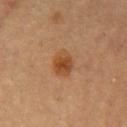The lesion's longest dimension is about 3.5 mm.
A female patient, aged around 60.
On the chest.
Automated tile analysis of the lesion measured an average lesion color of about L≈41 a*≈20 b*≈34 (CIELAB), roughly 9 lightness units darker than nearby skin, and a normalized lesion–skin contrast near 8.5. And it measured border irregularity of about 1.5 on a 0–10 scale, internal color variation of about 4 on a 0–10 scale, and a peripheral color-asymmetry measure near 1. And it measured a nevus-likeness score of about 95/100 and a lesion-detection confidence of about 100/100.
A 15 mm crop from a total-body photograph taken for skin-cancer surveillance.